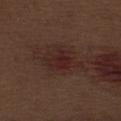follow-up — catalogued during a skin exam; not biopsied
location — the leg
diameter — ~5 mm (longest diameter)
subject — male, about 70 years old
image source — ~15 mm crop, total-body skin-cancer survey
TBP lesion metrics — an area of roughly 9.5 mm², an outline eccentricity of about 0.7 (0 = round, 1 = elongated), and two-axis asymmetry of about 0.4; roughly 6 lightness units darker than nearby skin and a normalized lesion–skin contrast near 7; a classifier nevus-likeness of about 5/100